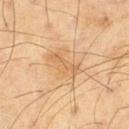biopsy status — no biopsy performed (imaged during a skin exam) | image — ~15 mm crop, total-body skin-cancer survey | patient — male, roughly 70 years of age | illumination — cross-polarized | lesion diameter — ≈4 mm | site — the right thigh | automated metrics — a lesion area of about 4.5 mm², a shape eccentricity near 0.95, and two-axis asymmetry of about 0.5; a detector confidence of about 100 out of 100 that the crop contains a lesion.